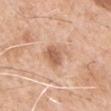workup = total-body-photography surveillance lesion; no biopsy
site = the left upper arm
lighting = white-light
acquisition = ~15 mm crop, total-body skin-cancer survey
patient = male, in their 60s